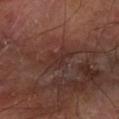No biopsy was performed on this lesion — it was imaged during a full skin examination and was not determined to be concerning. Automated tile analysis of the lesion measured border irregularity of about 4.5 on a 0–10 scale and a within-lesion color-variation index near 2/10. This image is a 15 mm lesion crop taken from a total-body photograph. About 3 mm across. On the left forearm. A male patient aged approximately 70.